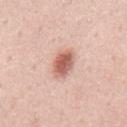Context: Automated tile analysis of the lesion measured an outline eccentricity of about 0.75 (0 = round, 1 = elongated). It also reported a border-irregularity index near 2/10, a within-lesion color-variation index near 4/10, and peripheral color asymmetry of about 1. Measured at roughly 3.5 mm in maximum diameter. Cropped from a total-body skin-imaging series; the visible field is about 15 mm. Imaged with white-light lighting. On the mid back. A male subject, roughly 55 years of age.